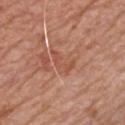follow-up = total-body-photography surveillance lesion; no biopsy | lighting = white-light illumination | image = total-body-photography crop, ~15 mm field of view | automated metrics = a symmetry-axis asymmetry near 0.55; a border-irregularity rating of about 7/10, a within-lesion color-variation index near 0/10, and peripheral color asymmetry of about 0; a nevus-likeness score of about 0/100 and lesion-presence confidence of about 75/100 | patient = male, in their 80s | body site = the chest.